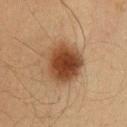Captured during whole-body skin photography for melanoma surveillance; the lesion was not biopsied.